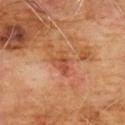notes = catalogued during a skin exam; not biopsied | subject = male, about 60 years old | image = 15 mm crop, total-body photography | lesion size = about 3 mm | body site = the chest | illumination = cross-polarized.